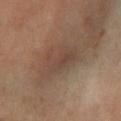Context:
The lesion-visualizer software estimated an eccentricity of roughly 0.8. It also reported a mean CIELAB color near L≈41 a*≈15 b*≈24, roughly 6 lightness units darker than nearby skin, and a normalized border contrast of about 5.5. The software also gave a border-irregularity rating of about 3.5/10, internal color variation of about 3 on a 0–10 scale, and radial color variation of about 1. A female subject aged 68–72. From the left lower leg. Approximately 5 mm at its widest. Cropped from a total-body skin-imaging series; the visible field is about 15 mm. Captured under cross-polarized illumination.